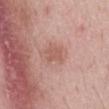anatomic site: the mid back
imaging modality: ~15 mm crop, total-body skin-cancer survey
TBP lesion metrics: a classifier nevus-likeness of about 0/100 and lesion-presence confidence of about 100/100
tile lighting: white-light
subject: male, aged around 65
size: about 2.5 mm